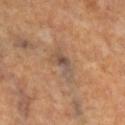Impression:
This lesion was catalogued during total-body skin photography and was not selected for biopsy.
Image and clinical context:
A female subject, roughly 65 years of age. The lesion is located on the right lower leg. A region of skin cropped from a whole-body photographic capture, roughly 15 mm wide.